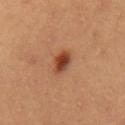The lesion was photographed on a routine skin check and not biopsied; there is no pathology result. A 15 mm close-up extracted from a 3D total-body photography capture. The patient is a female approximately 35 years of age. The lesion is located on the left upper arm.